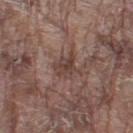Clinical impression:
Part of a total-body skin-imaging series; this lesion was reviewed on a skin check and was not flagged for biopsy.
Clinical summary:
The lesion-visualizer software estimated a mean CIELAB color near L≈41 a*≈17 b*≈21, a lesion–skin lightness drop of about 9, and a lesion-to-skin contrast of about 7 (normalized; higher = more distinct). The analysis additionally found border irregularity of about 4.5 on a 0–10 scale, a within-lesion color-variation index near 3.5/10, and radial color variation of about 1. The software also gave an automated nevus-likeness rating near 0 out of 100. A region of skin cropped from a whole-body photographic capture, roughly 15 mm wide. The subject is a male aged 78 to 82. Located on the right thigh. This is a white-light tile. Longest diameter approximately 3 mm.